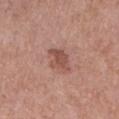Q: Is there a histopathology result?
A: catalogued during a skin exam; not biopsied
Q: How was this image acquired?
A: total-body-photography crop, ~15 mm field of view
Q: How large is the lesion?
A: ~3 mm (longest diameter)
Q: What is the anatomic site?
A: the right forearm
Q: Who is the patient?
A: female, aged approximately 40
Q: What lighting was used for the tile?
A: white-light